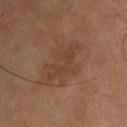Assessment: The lesion was tiled from a total-body skin photograph and was not biopsied. Clinical summary: Cropped from a total-body skin-imaging series; the visible field is about 15 mm. The lesion is on the upper back. A male patient, aged 38–42. This is a cross-polarized tile. Approximately 4.5 mm at its widest. Automated image analysis of the tile measured an area of roughly 7 mm², an eccentricity of roughly 0.85, and two-axis asymmetry of about 0.55. The analysis additionally found a nevus-likeness score of about 0/100 and a detector confidence of about 100 out of 100 that the crop contains a lesion.